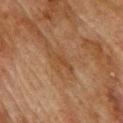Findings:
• notes · imaged on a skin check; not biopsied